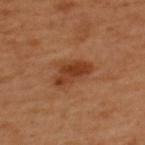biopsy_status: not biopsied; imaged during a skin examination